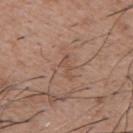This lesion was catalogued during total-body skin photography and was not selected for biopsy.
Captured under white-light illumination.
The recorded lesion diameter is about 3 mm.
From the back.
The total-body-photography lesion software estimated an outline eccentricity of about 0.9 (0 = round, 1 = elongated) and two-axis asymmetry of about 0.6. It also reported an average lesion color of about L≈50 a*≈19 b*≈28 (CIELAB), a lesion–skin lightness drop of about 6, and a normalized lesion–skin contrast near 4.5. The analysis additionally found border irregularity of about 6.5 on a 0–10 scale.
The subject is a male aged around 50.
A 15 mm close-up extracted from a 3D total-body photography capture.